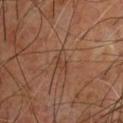A 15 mm crop from a total-body photograph taken for skin-cancer surveillance.
A male patient aged around 60.
On the upper back.
The tile uses cross-polarized illumination.
An algorithmic analysis of the crop reported a footprint of about 3.5 mm² and an eccentricity of roughly 0.8. It also reported a lesion–skin lightness drop of about 5 and a normalized lesion–skin contrast near 5. And it measured border irregularity of about 3.5 on a 0–10 scale and internal color variation of about 1.5 on a 0–10 scale. And it measured a nevus-likeness score of about 0/100 and lesion-presence confidence of about 95/100.